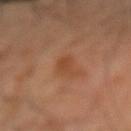Clinical impression: Part of a total-body skin-imaging series; this lesion was reviewed on a skin check and was not flagged for biopsy. Clinical summary: The total-body-photography lesion software estimated a lesion area of about 3.5 mm² and two-axis asymmetry of about 0.25. The software also gave a mean CIELAB color near L≈46 a*≈24 b*≈35, a lesion–skin lightness drop of about 7, and a lesion-to-skin contrast of about 6 (normalized; higher = more distinct). The software also gave a classifier nevus-likeness of about 0/100. On the right forearm. A lesion tile, about 15 mm wide, cut from a 3D total-body photograph. A male patient aged 63 to 67. The tile uses cross-polarized illumination. The recorded lesion diameter is about 2.5 mm.